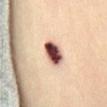Assessment: No biopsy was performed on this lesion — it was imaged during a full skin examination and was not determined to be concerning. Acquisition and patient details: A female patient aged 38 to 42. A region of skin cropped from a whole-body photographic capture, roughly 15 mm wide. Located on the abdomen.